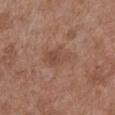Notes:
• notes · no biopsy performed (imaged during a skin exam)
• subject · female, roughly 80 years of age
• imaging modality · 15 mm crop, total-body photography
• diameter · about 4 mm
• illumination · white-light illumination
• location · the chest